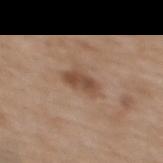{"biopsy_status": "not biopsied; imaged during a skin examination", "lesion_size": {"long_diameter_mm_approx": 4.0}, "site": "back", "image": {"source": "total-body photography crop", "field_of_view_mm": 15}, "lighting": "white-light", "patient": {"sex": "female", "age_approx": 65}}